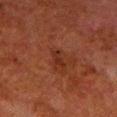Impression:
Imaged during a routine full-body skin examination; the lesion was not biopsied and no histopathology is available.